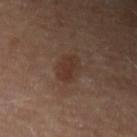{
  "biopsy_status": "not biopsied; imaged during a skin examination",
  "image": {
    "source": "total-body photography crop",
    "field_of_view_mm": 15
  },
  "patient": {
    "sex": "female",
    "age_approx": 45
  },
  "site": "left forearm"
}